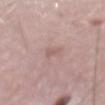Findings:
• biopsy status: catalogued during a skin exam; not biopsied
• subject: male, roughly 55 years of age
• lesion size: ~2.5 mm (longest diameter)
• image: total-body-photography crop, ~15 mm field of view
• tile lighting: white-light illumination
• automated metrics: a lesion-detection confidence of about 95/100